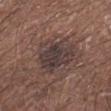{
  "biopsy_status": "not biopsied; imaged during a skin examination",
  "image": {
    "source": "total-body photography crop",
    "field_of_view_mm": 15
  },
  "patient": {
    "sex": "male",
    "age_approx": 65
  },
  "site": "left upper arm",
  "automated_metrics": {
    "area_mm2_approx": 19.0,
    "shape_asymmetry": 0.25,
    "cielab_L": 37,
    "cielab_a": 14,
    "cielab_b": 17,
    "vs_skin_darker_L": 8.0,
    "vs_skin_contrast_norm": 9.0
  },
  "lighting": "white-light",
  "lesion_size": {
    "long_diameter_mm_approx": 5.5
  }
}